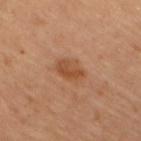Assessment: Imaged during a routine full-body skin examination; the lesion was not biopsied and no histopathology is available. Clinical summary: Cropped from a total-body skin-imaging series; the visible field is about 15 mm. A female patient, in their mid- to late 40s. From the right upper arm.